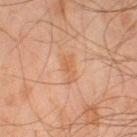From the left lower leg.
The subject is a male approximately 45 years of age.
Automated image analysis of the tile measured a lesion area of about 4 mm², an eccentricity of roughly 0.9, and a shape-asymmetry score of about 0.4 (0 = symmetric).
The lesion's longest dimension is about 3.5 mm.
A close-up tile cropped from a whole-body skin photograph, about 15 mm across.